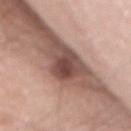Captured during whole-body skin photography for melanoma surveillance; the lesion was not biopsied. A close-up tile cropped from a whole-body skin photograph, about 15 mm across. The lesion's longest dimension is about 4.5 mm. The subject is a male aged 68–72. From the back. Captured under white-light illumination.